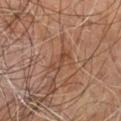Recorded during total-body skin imaging; not selected for excision or biopsy. Located on the right leg. A region of skin cropped from a whole-body photographic capture, roughly 15 mm wide. A male patient aged approximately 60.